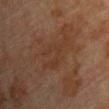<lesion>
  <biopsy_status>not biopsied; imaged during a skin examination</biopsy_status>
  <lighting>cross-polarized</lighting>
  <patient>
    <sex>male</sex>
    <age_approx>75</age_approx>
  </patient>
  <site>front of the torso</site>
  <lesion_size>
    <long_diameter_mm_approx>6.0</long_diameter_mm_approx>
  </lesion_size>
  <image>
    <source>total-body photography crop</source>
    <field_of_view_mm>15</field_of_view_mm>
  </image>
</lesion>